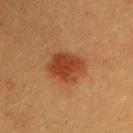Notes:
- follow-up: imaged on a skin check; not biopsied
- image: ~15 mm tile from a whole-body skin photo
- subject: male, in their 40s
- lesion size: ~4.5 mm (longest diameter)
- site: the right upper arm
- image-analysis metrics: an area of roughly 12 mm² and an eccentricity of roughly 0.6; a mean CIELAB color near L≈35 a*≈24 b*≈31, about 10 CIELAB-L* units darker than the surrounding skin, and a normalized border contrast of about 8.5; a border-irregularity rating of about 2/10 and a peripheral color-asymmetry measure near 1; a classifier nevus-likeness of about 100/100 and lesion-presence confidence of about 100/100
- lighting: cross-polarized